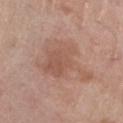Q: Was this lesion biopsied?
A: no biopsy performed (imaged during a skin exam)
Q: What lighting was used for the tile?
A: white-light
Q: How was this image acquired?
A: ~15 mm tile from a whole-body skin photo
Q: What are the patient's age and sex?
A: male, aged 68 to 72
Q: What is the anatomic site?
A: the left lower leg